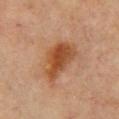subject = female, approximately 65 years of age; anatomic site = the chest; acquisition = ~15 mm tile from a whole-body skin photo; lesion size = ≈5.5 mm.